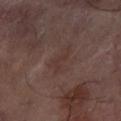The lesion was photographed on a routine skin check and not biopsied; there is no pathology result.
This image is a 15 mm lesion crop taken from a total-body photograph.
From the leg.
Automated image analysis of the tile measured an area of roughly 3.5 mm² and an outline eccentricity of about 0.9 (0 = round, 1 = elongated). The analysis additionally found a lesion color around L≈34 a*≈17 b*≈20 in CIELAB, about 4 CIELAB-L* units darker than the surrounding skin, and a normalized border contrast of about 5. And it measured an automated nevus-likeness rating near 0 out of 100.
Captured under cross-polarized illumination.